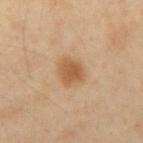Q: Is there a histopathology result?
A: no biopsy performed (imaged during a skin exam)
Q: Patient demographics?
A: male, aged 53–57
Q: What is the anatomic site?
A: the mid back
Q: Automated lesion metrics?
A: a border-irregularity rating of about 2.5/10 and a within-lesion color-variation index near 2/10; a classifier nevus-likeness of about 95/100
Q: What is the imaging modality?
A: total-body-photography crop, ~15 mm field of view
Q: How was the tile lit?
A: cross-polarized
Q: What is the lesion's diameter?
A: ~3 mm (longest diameter)